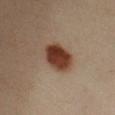| feature | finding |
|---|---|
| follow-up | total-body-photography surveillance lesion; no biopsy |
| size | about 5 mm |
| tile lighting | cross-polarized |
| patient | female, about 40 years old |
| body site | the left forearm |
| automated metrics | a footprint of about 12 mm², an outline eccentricity of about 0.85 (0 = round, 1 = elongated), and two-axis asymmetry of about 0.15; a classifier nevus-likeness of about 100/100 and lesion-presence confidence of about 100/100 |
| image | ~15 mm tile from a whole-body skin photo |